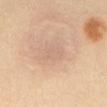Clinical impression: Part of a total-body skin-imaging series; this lesion was reviewed on a skin check and was not flagged for biopsy. Acquisition and patient details: Automated image analysis of the tile measured a nevus-likeness score of about 0/100. A 15 mm close-up tile from a total-body photography series done for melanoma screening. The tile uses cross-polarized illumination. The lesion is located on the abdomen. Longest diameter approximately 3 mm. A female subject aged 23 to 27.